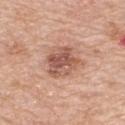{"biopsy_status": "not biopsied; imaged during a skin examination", "site": "back", "image": {"source": "total-body photography crop", "field_of_view_mm": 15}, "patient": {"sex": "female", "age_approx": 65}}